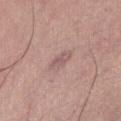biopsy status: imaged on a skin check; not biopsied | image-analysis metrics: a shape eccentricity near 0.9 and two-axis asymmetry of about 0.2; an average lesion color of about L≈56 a*≈19 b*≈20 (CIELAB), roughly 8 lightness units darker than nearby skin, and a lesion-to-skin contrast of about 5.5 (normalized; higher = more distinct); internal color variation of about 1.5 on a 0–10 scale and peripheral color asymmetry of about 0.5 | subject: female, in their mid- to late 40s | site: the front of the torso | lighting: white-light illumination | acquisition: ~15 mm crop, total-body skin-cancer survey.